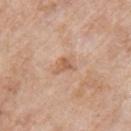{"site": "left upper arm", "lighting": "white-light", "patient": {"sex": "female", "age_approx": 70}, "image": {"source": "total-body photography crop", "field_of_view_mm": 15}}